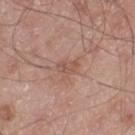notes=no biopsy performed (imaged during a skin exam)
site=the left thigh
acquisition=15 mm crop, total-body photography
subject=male, in their mid- to late 50s